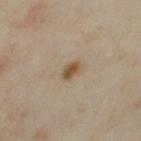The lesion was photographed on a routine skin check and not biopsied; there is no pathology result. A female patient, about 35 years old. On the left upper arm. A close-up tile cropped from a whole-body skin photograph, about 15 mm across.